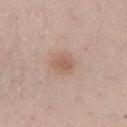{"biopsy_status": "not biopsied; imaged during a skin examination"}